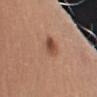Assessment: Recorded during total-body skin imaging; not selected for excision or biopsy. Acquisition and patient details: The subject is a male about 55 years old. Approximately 6 mm at its widest. Cropped from a total-body skin-imaging series; the visible field is about 15 mm. From the left upper arm. Automated image analysis of the tile measured an area of roughly 12 mm², a shape eccentricity near 0.9, and a shape-asymmetry score of about 0.25 (0 = symmetric). The software also gave a lesion–skin lightness drop of about 7 and a lesion-to-skin contrast of about 5 (normalized; higher = more distinct). It also reported a border-irregularity rating of about 3.5/10, internal color variation of about 9.5 on a 0–10 scale, and peripheral color asymmetry of about 4.